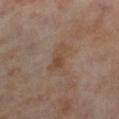Impression: Imaged during a routine full-body skin examination; the lesion was not biopsied and no histopathology is available. Background: Approximately 4.5 mm at its widest. Located on the left lower leg. A female subject approximately 55 years of age. A 15 mm close-up extracted from a 3D total-body photography capture.